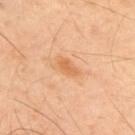Clinical impression: Recorded during total-body skin imaging; not selected for excision or biopsy. Context: The subject is a male approximately 60 years of age. A lesion tile, about 15 mm wide, cut from a 3D total-body photograph. From the upper back. The tile uses cross-polarized illumination. Longest diameter approximately 2.5 mm.